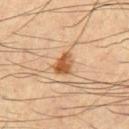notes=catalogued during a skin exam; not biopsied
imaging modality=~15 mm tile from a whole-body skin photo
patient=male, aged approximately 60